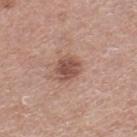{"biopsy_status": "not biopsied; imaged during a skin examination", "patient": {"sex": "male", "age_approx": 50}, "image": {"source": "total-body photography crop", "field_of_view_mm": 15}, "lesion_size": {"long_diameter_mm_approx": 3.0}, "site": "right lower leg", "automated_metrics": {"area_mm2_approx": 6.5, "eccentricity": 0.5, "border_irregularity_0_10": 2.0, "color_variation_0_10": 3.0, "peripheral_color_asymmetry": 1.0, "nevus_likeness_0_100": 70, "lesion_detection_confidence_0_100": 100}}